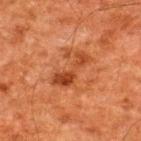No biopsy was performed on this lesion — it was imaged during a full skin examination and was not determined to be concerning. The lesion is on the upper back. Cropped from a whole-body photographic skin survey; the tile spans about 15 mm. A male subject, aged 58 to 62.